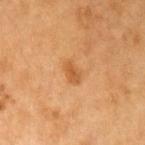automated_metrics:
  cielab_L: 57
  cielab_a: 25
  cielab_b: 43
  vs_skin_darker_L: 9.0
  vs_skin_contrast_norm: 6.5
  border_irregularity_0_10: 2.0
  peripheral_color_asymmetry: 1.0
site: left upper arm
lesion_size:
  long_diameter_mm_approx: 2.5
lighting: cross-polarized
patient:
  sex: male
  age_approx: 60
image:
  source: total-body photography crop
  field_of_view_mm: 15